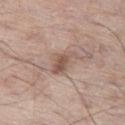Imaged during a routine full-body skin examination; the lesion was not biopsied and no histopathology is available. The lesion is located on the left thigh. The total-body-photography lesion software estimated a lesion area of about 6 mm², a shape eccentricity near 0.85, and a shape-asymmetry score of about 0.4 (0 = symmetric). It also reported a within-lesion color-variation index near 3/10 and a peripheral color-asymmetry measure near 1. The lesion's longest dimension is about 4 mm. A close-up tile cropped from a whole-body skin photograph, about 15 mm across. The patient is a male aged 63–67. This is a white-light tile.